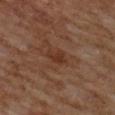{"lesion_size": {"long_diameter_mm_approx": 3.0}, "patient": {"sex": "female", "age_approx": 60}, "lighting": "cross-polarized", "image": {"source": "total-body photography crop", "field_of_view_mm": 15}, "automated_metrics": {"border_irregularity_0_10": 3.5, "color_variation_0_10": 1.5, "peripheral_color_asymmetry": 0.5}, "site": "upper back"}